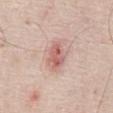Clinical impression:
No biopsy was performed on this lesion — it was imaged during a full skin examination and was not determined to be concerning.
Clinical summary:
A male patient approximately 70 years of age. Captured under white-light illumination. An algorithmic analysis of the crop reported a lesion area of about 7.5 mm², an eccentricity of roughly 0.75, and a shape-asymmetry score of about 0.25 (0 = symmetric). And it measured a mean CIELAB color near L≈62 a*≈24 b*≈25 and roughly 11 lightness units darker than nearby skin. The analysis additionally found border irregularity of about 2.5 on a 0–10 scale, a color-variation rating of about 4.5/10, and a peripheral color-asymmetry measure near 1.5. A region of skin cropped from a whole-body photographic capture, roughly 15 mm wide. Located on the abdomen. The recorded lesion diameter is about 4 mm.